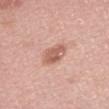workup: imaged on a skin check; not biopsied.